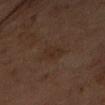Imaged during a routine full-body skin examination; the lesion was not biopsied and no histopathology is available. A female subject, approximately 60 years of age. Cropped from a total-body skin-imaging series; the visible field is about 15 mm. The lesion is located on the right upper arm. The lesion-visualizer software estimated a mean CIELAB color near L≈23 a*≈13 b*≈21 and a normalized lesion–skin contrast near 5. And it measured an automated nevus-likeness rating near 0 out of 100 and a detector confidence of about 100 out of 100 that the crop contains a lesion. This is a cross-polarized tile.